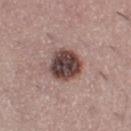* follow-up — catalogued during a skin exam; not biopsied
* lesion diameter — ≈4 mm
* lighting — white-light illumination
* automated lesion analysis — a footprint of about 12 mm² and a shape-asymmetry score of about 0.15 (0 = symmetric); a border-irregularity rating of about 1.5/10
* body site — the left thigh
* imaging modality — ~15 mm crop, total-body skin-cancer survey
* subject — female, aged around 55